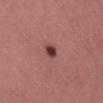The lesion was photographed on a routine skin check and not biopsied; there is no pathology result.
The patient is a female approximately 45 years of age.
Located on the right thigh.
A 15 mm close-up tile from a total-body photography series done for melanoma screening.
This is a white-light tile.